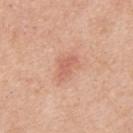Assessment:
The lesion was photographed on a routine skin check and not biopsied; there is no pathology result.
Clinical summary:
The lesion-visualizer software estimated a footprint of about 4 mm² and a shape-asymmetry score of about 0.5 (0 = symmetric). The software also gave a lesion color around L≈62 a*≈26 b*≈31 in CIELAB, roughly 8 lightness units darker than nearby skin, and a lesion-to-skin contrast of about 5.5 (normalized; higher = more distinct). It also reported border irregularity of about 5 on a 0–10 scale and peripheral color asymmetry of about 0.5. The software also gave an automated nevus-likeness rating near 15 out of 100 and a lesion-detection confidence of about 100/100. A male patient about 55 years old. A 15 mm crop from a total-body photograph taken for skin-cancer surveillance. About 3.5 mm across. From the mid back. Imaged with white-light lighting.